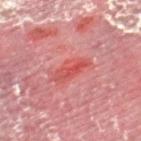The lesion was tiled from a total-body skin photograph and was not biopsied. Imaged with cross-polarized lighting. Cropped from a total-body skin-imaging series; the visible field is about 15 mm. Approximately 4.5 mm at its widest. The lesion-visualizer software estimated a color-variation rating of about 6.5/10. Located on the right lower leg. A male subject about 55 years old.